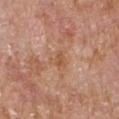Assessment:
This lesion was catalogued during total-body skin photography and was not selected for biopsy.
Background:
Cropped from a total-body skin-imaging series; the visible field is about 15 mm. An algorithmic analysis of the crop reported a border-irregularity rating of about 4/10. The software also gave a nevus-likeness score of about 0/100 and lesion-presence confidence of about 100/100. The lesion is located on the right upper arm. The lesion's longest dimension is about 3 mm. The patient is a male approximately 65 years of age.